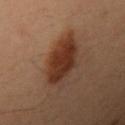workup=no biopsy performed (imaged during a skin exam) | subject=female, roughly 30 years of age | anatomic site=the right upper arm | image source=~15 mm tile from a whole-body skin photo.